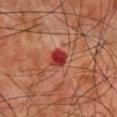Captured during whole-body skin photography for melanoma surveillance; the lesion was not biopsied. A 15 mm crop from a total-body photograph taken for skin-cancer surveillance. A male subject approximately 60 years of age. The lesion is located on the back. The lesion-visualizer software estimated an outline eccentricity of about 0.55 (0 = round, 1 = elongated) and two-axis asymmetry of about 0.2. The software also gave a mean CIELAB color near L≈36 a*≈35 b*≈28 and a normalized border contrast of about 10. It also reported a nevus-likeness score of about 0/100.